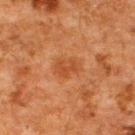Q: Was a biopsy performed?
A: no biopsy performed (imaged during a skin exam)
Q: Where on the body is the lesion?
A: the upper back
Q: What did automated image analysis measure?
A: about 6 CIELAB-L* units darker than the surrounding skin and a lesion-to-skin contrast of about 5.5 (normalized; higher = more distinct); a border-irregularity index near 2/10, a color-variation rating of about 2/10, and peripheral color asymmetry of about 1
Q: What is the imaging modality?
A: total-body-photography crop, ~15 mm field of view
Q: How was the tile lit?
A: cross-polarized
Q: What are the patient's age and sex?
A: male, aged approximately 60
Q: Lesion size?
A: ≈3.5 mm